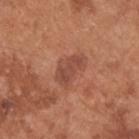Q: Was a biopsy performed?
A: no biopsy performed (imaged during a skin exam)
Q: What is the lesion's diameter?
A: about 4 mm
Q: How was this image acquired?
A: total-body-photography crop, ~15 mm field of view
Q: Who is the patient?
A: male, roughly 55 years of age
Q: Where on the body is the lesion?
A: the back
Q: What lighting was used for the tile?
A: white-light